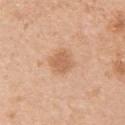Imaged during a routine full-body skin examination; the lesion was not biopsied and no histopathology is available. A female patient, aged around 40. The lesion's longest dimension is about 3 mm. Captured under white-light illumination. A 15 mm close-up tile from a total-body photography series done for melanoma screening. An algorithmic analysis of the crop reported a lesion area of about 6 mm², a shape eccentricity near 0.5, and a shape-asymmetry score of about 0.2 (0 = symmetric). The lesion is on the upper back.